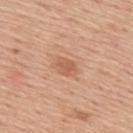workup=imaged on a skin check; not biopsied
imaging modality=~15 mm crop, total-body skin-cancer survey
body site=the upper back
patient=male, approximately 60 years of age
tile lighting=white-light illumination
lesion diameter=≈3 mm